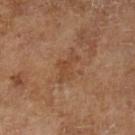Q: Was a biopsy performed?
A: catalogued during a skin exam; not biopsied
Q: How was this image acquired?
A: ~15 mm crop, total-body skin-cancer survey
Q: Automated lesion metrics?
A: an area of roughly 7 mm² and an eccentricity of roughly 0.8; a lesion color around L≈44 a*≈20 b*≈32 in CIELAB and a lesion–skin lightness drop of about 6; a detector confidence of about 100 out of 100 that the crop contains a lesion
Q: Patient demographics?
A: male, in their mid-60s
Q: How was the tile lit?
A: cross-polarized